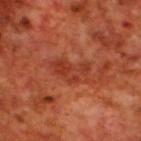This lesion was catalogued during total-body skin photography and was not selected for biopsy. The recorded lesion diameter is about 4.5 mm. A male subject approximately 70 years of age. Located on the upper back. A 15 mm close-up extracted from a 3D total-body photography capture.